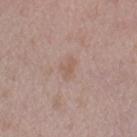Captured during whole-body skin photography for melanoma surveillance; the lesion was not biopsied. The patient is a male aged 48 to 52. A roughly 15 mm field-of-view crop from a total-body skin photograph. The lesion is on the left upper arm.